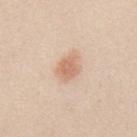No biopsy was performed on this lesion — it was imaged during a full skin examination and was not determined to be concerning.
A male patient aged approximately 25.
Located on the mid back.
This image is a 15 mm lesion crop taken from a total-body photograph.